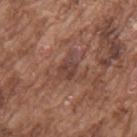Findings:
* follow-up · total-body-photography surveillance lesion; no biopsy
* body site · the upper back
* subject · male, aged 73 to 77
* size · ≈3 mm
* image-analysis metrics · an area of roughly 5 mm² and a symmetry-axis asymmetry near 0.3; border irregularity of about 3.5 on a 0–10 scale, a within-lesion color-variation index near 2.5/10, and peripheral color asymmetry of about 1
* acquisition · 15 mm crop, total-body photography
* lighting · white-light